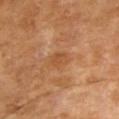follow-up: imaged on a skin check; not biopsied
body site: the right upper arm
imaging modality: ~15 mm crop, total-body skin-cancer survey
diameter: about 2.5 mm
illumination: cross-polarized illumination
subject: female, about 70 years old